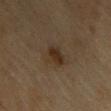Clinical summary:
A female patient approximately 60 years of age. An algorithmic analysis of the crop reported a footprint of about 5.5 mm², a shape eccentricity near 0.45, and a shape-asymmetry score of about 0.35 (0 = symmetric). And it measured a mean CIELAB color near L≈27 a*≈12 b*≈24, roughly 7 lightness units darker than nearby skin, and a normalized border contrast of about 8. The software also gave a border-irregularity index near 3/10, internal color variation of about 2.5 on a 0–10 scale, and peripheral color asymmetry of about 1. The analysis additionally found a classifier nevus-likeness of about 65/100 and lesion-presence confidence of about 100/100. A region of skin cropped from a whole-body photographic capture, roughly 15 mm wide. The lesion is on the front of the torso. Measured at roughly 2.5 mm in maximum diameter. Captured under cross-polarized illumination.